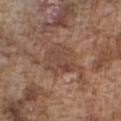The lesion was tiled from a total-body skin photograph and was not biopsied.
A male subject in their mid-70s.
Imaged with white-light lighting.
The lesion is located on the front of the torso.
This image is a 15 mm lesion crop taken from a total-body photograph.
The total-body-photography lesion software estimated a footprint of about 9.5 mm² and a symmetry-axis asymmetry near 0.25. The software also gave an average lesion color of about L≈45 a*≈18 b*≈27 (CIELAB), roughly 7 lightness units darker than nearby skin, and a normalized lesion–skin contrast near 5.5. The analysis additionally found a nevus-likeness score of about 5/100 and lesion-presence confidence of about 100/100.